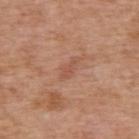No biopsy was performed on this lesion — it was imaged during a full skin examination and was not determined to be concerning.
This is a white-light tile.
The lesion is on the back.
A 15 mm crop from a total-body photograph taken for skin-cancer surveillance.
Approximately 3 mm at its widest.
The patient is a male aged around 60.